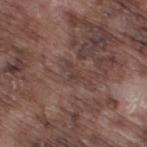The subject is a male approximately 75 years of age. This is a white-light tile. Cropped from a whole-body photographic skin survey; the tile spans about 15 mm. Automated image analysis of the tile measured a footprint of about 2.5 mm², an eccentricity of roughly 0.85, and a shape-asymmetry score of about 0.4 (0 = symmetric). Measured at roughly 2.5 mm in maximum diameter. Located on the leg.